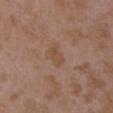Imaged during a routine full-body skin examination; the lesion was not biopsied and no histopathology is available. A female patient, roughly 35 years of age. An algorithmic analysis of the crop reported a border-irregularity rating of about 3/10 and internal color variation of about 1 on a 0–10 scale. The analysis additionally found an automated nevus-likeness rating near 0 out of 100. A 15 mm crop from a total-body photograph taken for skin-cancer surveillance. Measured at roughly 3 mm in maximum diameter. This is a white-light tile. On the left upper arm.